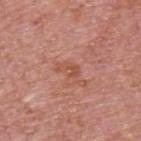| field | value |
|---|---|
| patient | male, aged 73–77 |
| automated metrics | a footprint of about 2.5 mm² and an outline eccentricity of about 0.8 (0 = round, 1 = elongated); an average lesion color of about L≈52 a*≈26 b*≈31 (CIELAB), about 7 CIELAB-L* units darker than the surrounding skin, and a normalized lesion–skin contrast near 5.5; a border-irregularity index near 6.5/10, internal color variation of about 0 on a 0–10 scale, and peripheral color asymmetry of about 0 |
| image source | total-body-photography crop, ~15 mm field of view |
| tile lighting | white-light |
| size | ~2.5 mm (longest diameter) |
| anatomic site | the upper back |